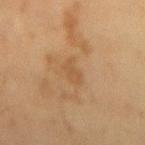No biopsy was performed on this lesion — it was imaged during a full skin examination and was not determined to be concerning. A 15 mm close-up tile from a total-body photography series done for melanoma screening. The total-body-photography lesion software estimated an automated nevus-likeness rating near 0 out of 100 and lesion-presence confidence of about 100/100. Captured under cross-polarized illumination. A male subject, approximately 60 years of age. On the lower back. The lesion's longest dimension is about 3 mm.